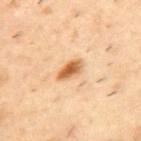<case>
  <biopsy_status>not biopsied; imaged during a skin examination</biopsy_status>
</case>